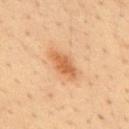Clinical impression: Captured during whole-body skin photography for melanoma surveillance; the lesion was not biopsied. Image and clinical context: A 15 mm close-up extracted from a 3D total-body photography capture. A male subject, approximately 35 years of age. Located on the back.